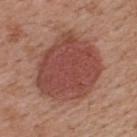Q: Is there a histopathology result?
A: catalogued during a skin exam; not biopsied
Q: What are the patient's age and sex?
A: male, aged 53 to 57
Q: How was this image acquired?
A: 15 mm crop, total-body photography
Q: Lesion location?
A: the upper back
Q: What did automated image analysis measure?
A: an area of roughly 43 mm², an eccentricity of roughly 0.5, and two-axis asymmetry of about 0.15; an average lesion color of about L≈46 a*≈25 b*≈26 (CIELAB) and about 11 CIELAB-L* units darker than the surrounding skin; a nevus-likeness score of about 100/100 and lesion-presence confidence of about 100/100